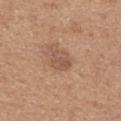An algorithmic analysis of the crop reported a classifier nevus-likeness of about 10/100.
The lesion is located on the mid back.
This is a white-light tile.
A male patient roughly 65 years of age.
A close-up tile cropped from a whole-body skin photograph, about 15 mm across.
Approximately 2.5 mm at its widest.